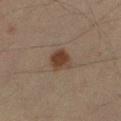Measured at roughly 3 mm in maximum diameter. This is a cross-polarized tile. Automated image analysis of the tile measured a lesion area of about 6 mm² and a symmetry-axis asymmetry near 0.2. A male patient approximately 35 years of age. This image is a 15 mm lesion crop taken from a total-body photograph. The lesion is located on the right forearm.